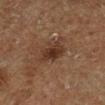| field | value |
|---|---|
| biopsy status | total-body-photography surveillance lesion; no biopsy |
| imaging modality | ~15 mm crop, total-body skin-cancer survey |
| subject | male, aged around 75 |
| tile lighting | cross-polarized illumination |
| site | the right lower leg |